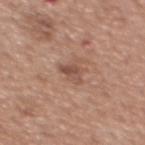{
  "biopsy_status": "not biopsied; imaged during a skin examination",
  "site": "back",
  "lighting": "white-light",
  "patient": {
    "sex": "female",
    "age_approx": 50
  },
  "lesion_size": {
    "long_diameter_mm_approx": 2.5
  },
  "image": {
    "source": "total-body photography crop",
    "field_of_view_mm": 15
  }
}